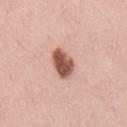The lesion was photographed on a routine skin check and not biopsied; there is no pathology result.
Located on the mid back.
The patient is a male aged approximately 35.
A lesion tile, about 15 mm wide, cut from a 3D total-body photograph.